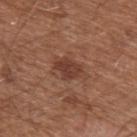Impression:
No biopsy was performed on this lesion — it was imaged during a full skin examination and was not determined to be concerning.
Context:
Longest diameter approximately 3 mm. A 15 mm close-up extracted from a 3D total-body photography capture. The total-body-photography lesion software estimated an area of roughly 5.5 mm², an outline eccentricity of about 0.7 (0 = round, 1 = elongated), and a symmetry-axis asymmetry near 0.25. The analysis additionally found a classifier nevus-likeness of about 60/100 and a detector confidence of about 100 out of 100 that the crop contains a lesion. Captured under white-light illumination. A male subject, about 65 years old. The lesion is located on the arm.